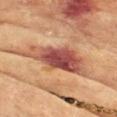Clinical impression: Imaged during a routine full-body skin examination; the lesion was not biopsied and no histopathology is available. Context: This is a cross-polarized tile. Located on the front of the torso. Approximately 8 mm at its widest. The subject is a female about 80 years old. A 15 mm close-up extracted from a 3D total-body photography capture.